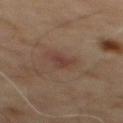<lesion>
<lesion_size>
  <long_diameter_mm_approx>2.5</long_diameter_mm_approx>
</lesion_size>
<lighting>cross-polarized</lighting>
<image>
  <source>total-body photography crop</source>
  <field_of_view_mm>15</field_of_view_mm>
</image>
<automated_metrics>
  <border_irregularity_0_10>3.5</border_irregularity_0_10>
  <color_variation_0_10>1.5</color_variation_0_10>
  <peripheral_color_asymmetry>0.5</peripheral_color_asymmetry>
</automated_metrics>
<patient>
  <sex>male</sex>
  <age_approx>65</age_approx>
</patient>
<site>mid back</site>
</lesion>